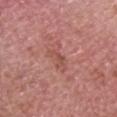Q: Was this lesion biopsied?
A: total-body-photography surveillance lesion; no biopsy
Q: Lesion location?
A: the head or neck
Q: Illumination type?
A: white-light
Q: Lesion size?
A: about 3 mm
Q: What kind of image is this?
A: 15 mm crop, total-body photography
Q: Patient demographics?
A: male, aged approximately 75